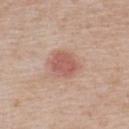workup=catalogued during a skin exam; not biopsied
automated lesion analysis=a lesion color around L≈57 a*≈25 b*≈26 in CIELAB, roughly 11 lightness units darker than nearby skin, and a normalized border contrast of about 7; a border-irregularity rating of about 1.5/10, a color-variation rating of about 2.5/10, and radial color variation of about 1; a nevus-likeness score of about 85/100
body site=the upper back
size=about 4 mm
patient=male, in their mid- to late 70s
tile lighting=white-light illumination
imaging modality=~15 mm tile from a whole-body skin photo